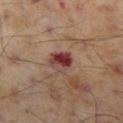The lesion was tiled from a total-body skin photograph and was not biopsied. The lesion's longest dimension is about 3 mm. The lesion is on the left thigh. A male patient, in their mid-50s. Cropped from a total-body skin-imaging series; the visible field is about 15 mm. The tile uses cross-polarized illumination.